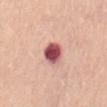  biopsy_status: not biopsied; imaged during a skin examination
  image:
    source: total-body photography crop
    field_of_view_mm: 15
  site: lower back
  lighting: white-light
  patient:
    sex: female
    age_approx: 65